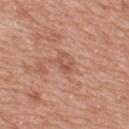<tbp_lesion>
<image>
  <source>total-body photography crop</source>
  <field_of_view_mm>15</field_of_view_mm>
</image>
<automated_metrics>
  <eccentricity>0.9</eccentricity>
  <shape_asymmetry>0.5</shape_asymmetry>
  <cielab_L>54</cielab_L>
  <cielab_a>23</cielab_a>
  <cielab_b>31</cielab_b>
  <vs_skin_contrast_norm>5.5</vs_skin_contrast_norm>
  <border_irregularity_0_10>6.0</border_irregularity_0_10>
  <color_variation_0_10>0.0</color_variation_0_10>
  <peripheral_color_asymmetry>0.0</peripheral_color_asymmetry>
  <nevus_likeness_0_100>5</nevus_likeness_0_100>
  <lesion_detection_confidence_0_100>100</lesion_detection_confidence_0_100>
</automated_metrics>
<lighting>white-light</lighting>
<patient>
  <sex>male</sex>
  <age_approx>50</age_approx>
</patient>
<site>mid back</site>
</tbp_lesion>